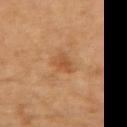Context:
Captured under cross-polarized illumination. From the left forearm. This image is a 15 mm lesion crop taken from a total-body photograph. Automated tile analysis of the lesion measured an outline eccentricity of about 0.55 (0 = round, 1 = elongated). The software also gave a lesion–skin lightness drop of about 8. The analysis additionally found border irregularity of about 3 on a 0–10 scale, a color-variation rating of about 2/10, and radial color variation of about 0.5. A female subject aged around 65.